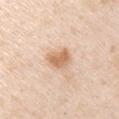Recorded during total-body skin imaging; not selected for excision or biopsy. The patient is a male aged around 45. On the left upper arm. A roughly 15 mm field-of-view crop from a total-body skin photograph.